Captured under white-light illumination · on the head or neck · cropped from a whole-body photographic skin survey; the tile spans about 15 mm · about 9 mm across · Automated image analysis of the tile measured border irregularity of about 5 on a 0–10 scale and a color-variation rating of about 7/10 · a male subject, aged 48 to 52 — 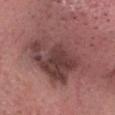Q: What did pathology find?
A: a lentigo